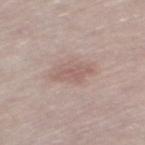Impression: The lesion was tiled from a total-body skin photograph and was not biopsied. Clinical summary: A male patient aged around 65. A close-up tile cropped from a whole-body skin photograph, about 15 mm across. Located on the left thigh. The recorded lesion diameter is about 4 mm.